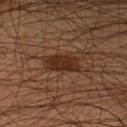The lesion was tiled from a total-body skin photograph and was not biopsied.
The lesion's longest dimension is about 5 mm.
The subject is a male aged approximately 45.
The lesion is located on the left lower leg.
A lesion tile, about 15 mm wide, cut from a 3D total-body photograph.
Automated image analysis of the tile measured a footprint of about 9 mm², an eccentricity of roughly 0.9, and a shape-asymmetry score of about 0.25 (0 = symmetric). It also reported a lesion color around L≈28 a*≈18 b*≈26 in CIELAB, about 9 CIELAB-L* units darker than the surrounding skin, and a lesion-to-skin contrast of about 9.5 (normalized; higher = more distinct). The software also gave a nevus-likeness score of about 85/100 and a lesion-detection confidence of about 100/100.